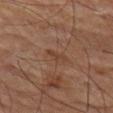About 2.5 mm across. Cropped from a total-body skin-imaging series; the visible field is about 15 mm. The lesion is on the left thigh. The tile uses cross-polarized illumination. A male subject about 65 years old.